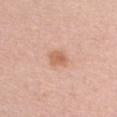Clinical impression:
Imaged during a routine full-body skin examination; the lesion was not biopsied and no histopathology is available.
Background:
The lesion is located on the chest. A female patient, aged around 40. Approximately 3 mm at its widest. Captured under white-light illumination. The total-body-photography lesion software estimated a footprint of about 5 mm² and two-axis asymmetry of about 0.25. A lesion tile, about 15 mm wide, cut from a 3D total-body photograph.